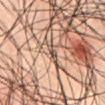workup=imaged on a skin check; not biopsied
patient=male, in their mid-40s
anatomic site=the abdomen
automated metrics=two-axis asymmetry of about 0.25; a mean CIELAB color near L≈49 a*≈16 b*≈25, roughly 12 lightness units darker than nearby skin, and a normalized lesion–skin contrast near 9; border irregularity of about 2.5 on a 0–10 scale, a within-lesion color-variation index near 0/10, and radial color variation of about 0
imaging modality=15 mm crop, total-body photography
illumination=cross-polarized